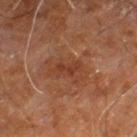{"biopsy_status": "not biopsied; imaged during a skin examination", "site": "right leg", "patient": {"sex": "male", "age_approx": 60}, "lesion_size": {"long_diameter_mm_approx": 3.0}, "image": {"source": "total-body photography crop", "field_of_view_mm": 15}}